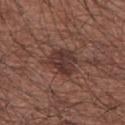<lesion>
<biopsy_status>not biopsied; imaged during a skin examination</biopsy_status>
<site>arm</site>
<lesion_size>
  <long_diameter_mm_approx>4.0</long_diameter_mm_approx>
</lesion_size>
<image>
  <source>total-body photography crop</source>
  <field_of_view_mm>15</field_of_view_mm>
</image>
<patient>
  <sex>male</sex>
  <age_approx>65</age_approx>
</patient>
<lighting>white-light</lighting>
<automated_metrics>
  <border_irregularity_0_10>3.5</border_irregularity_0_10>
  <color_variation_0_10>3.5</color_variation_0_10>
</automated_metrics>
</lesion>